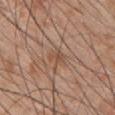Captured under white-light illumination. The lesion-visualizer software estimated an area of roughly 3 mm². And it measured border irregularity of about 3.5 on a 0–10 scale. The analysis additionally found lesion-presence confidence of about 70/100. Located on the chest. Cropped from a whole-body photographic skin survey; the tile spans about 15 mm. Measured at roughly 2.5 mm in maximum diameter. A male subject aged 48 to 52.